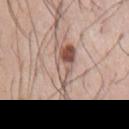  biopsy_status: not biopsied; imaged during a skin examination
  patient:
    sex: male
    age_approx: 75
  lesion_size:
    long_diameter_mm_approx: 6.0
  lighting: white-light
  site: chest
  image:
    source: total-body photography crop
    field_of_view_mm: 15